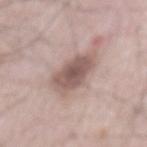{
  "biopsy_status": "not biopsied; imaged during a skin examination",
  "site": "mid back",
  "lesion_size": {
    "long_diameter_mm_approx": 6.0
  },
  "patient": {
    "sex": "male",
    "age_approx": 65
  },
  "image": {
    "source": "total-body photography crop",
    "field_of_view_mm": 15
  }
}